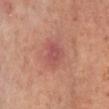biopsy status=total-body-photography surveillance lesion; no biopsy | acquisition=~15 mm crop, total-body skin-cancer survey | illumination=cross-polarized illumination | patient=female, aged 48–52 | location=the left lower leg.